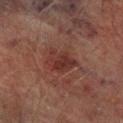biopsy_status: not biopsied; imaged during a skin examination
site: right lower leg
image:
  source: total-body photography crop
  field_of_view_mm: 15
patient:
  sex: male
  age_approx: 75